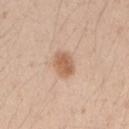workup: catalogued during a skin exam; not biopsied
imaging modality: total-body-photography crop, ~15 mm field of view
subject: female, in their mid-20s
automated lesion analysis: a classifier nevus-likeness of about 95/100 and lesion-presence confidence of about 100/100
location: the right upper arm